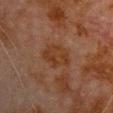follow-up=imaged on a skin check; not biopsied | patient=male, aged 78 to 82 | lighting=cross-polarized illumination | imaging modality=total-body-photography crop, ~15 mm field of view | anatomic site=the front of the torso | lesion diameter=≈4 mm.